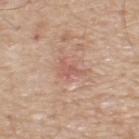Impression:
The lesion was photographed on a routine skin check and not biopsied; there is no pathology result.
Acquisition and patient details:
Measured at roughly 2.5 mm in maximum diameter. The patient is a male in their 70s. Cropped from a total-body skin-imaging series; the visible field is about 15 mm. The lesion is on the upper back. An algorithmic analysis of the crop reported an area of roughly 3 mm² and a shape-asymmetry score of about 0.45 (0 = symmetric). The analysis additionally found a mean CIELAB color near L≈58 a*≈23 b*≈27 and roughly 7 lightness units darker than nearby skin.